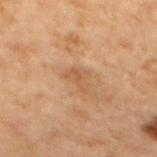Clinical impression:
Imaged during a routine full-body skin examination; the lesion was not biopsied and no histopathology is available.
Clinical summary:
A 15 mm crop from a total-body photograph taken for skin-cancer surveillance. On the mid back. A female subject, in their 60s.